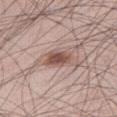Q: What is the anatomic site?
A: the right thigh
Q: How was the tile lit?
A: white-light illumination
Q: How large is the lesion?
A: ≈3.5 mm
Q: What is the imaging modality?
A: total-body-photography crop, ~15 mm field of view
Q: Patient demographics?
A: male, aged 43 to 47